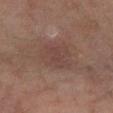{
  "biopsy_status": "not biopsied; imaged during a skin examination",
  "site": "left lower leg",
  "image": {
    "source": "total-body photography crop",
    "field_of_view_mm": 15
  },
  "lighting": "cross-polarized",
  "lesion_size": {
    "long_diameter_mm_approx": 4.0
  },
  "patient": {
    "sex": "female",
    "age_approx": 60
  }
}